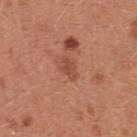{"lighting": "white-light", "automated_metrics": {"area_mm2_approx": 3.5, "eccentricity": 0.8, "shape_asymmetry": 0.25, "cielab_L": 49, "cielab_a": 27, "cielab_b": 32, "vs_skin_darker_L": 8.0, "vs_skin_contrast_norm": 6.0, "border_irregularity_0_10": 2.5, "color_variation_0_10": 1.0, "peripheral_color_asymmetry": 0.5}, "site": "chest", "lesion_size": {"long_diameter_mm_approx": 2.5}, "patient": {"sex": "female", "age_approx": 35}, "image": {"source": "total-body photography crop", "field_of_view_mm": 15}}